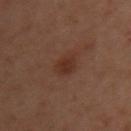biopsy_status: not biopsied; imaged during a skin examination
site: chest
patient:
  sex: female
  age_approx: 55
lesion_size:
  long_diameter_mm_approx: 3.0
image:
  source: total-body photography crop
  field_of_view_mm: 15